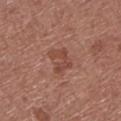No biopsy was performed on this lesion — it was imaged during a full skin examination and was not determined to be concerning. The tile uses white-light illumination. A 15 mm crop from a total-body photograph taken for skin-cancer surveillance. The lesion's longest dimension is about 3.5 mm. The total-body-photography lesion software estimated an average lesion color of about L≈46 a*≈23 b*≈27 (CIELAB), a lesion–skin lightness drop of about 8, and a lesion-to-skin contrast of about 6 (normalized; higher = more distinct). The software also gave a lesion-detection confidence of about 100/100. A male patient roughly 75 years of age. The lesion is located on the left lower leg.